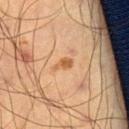| key | value |
|---|---|
| workup | no biopsy performed (imaged during a skin exam) |
| subject | male, aged 63 to 67 |
| diameter | about 2.5 mm |
| lighting | cross-polarized |
| anatomic site | the right thigh |
| acquisition | total-body-photography crop, ~15 mm field of view |